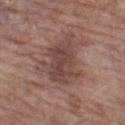Q: Was a biopsy performed?
A: catalogued during a skin exam; not biopsied
Q: Illumination type?
A: white-light illumination
Q: What is the anatomic site?
A: the leg
Q: What is the imaging modality?
A: ~15 mm crop, total-body skin-cancer survey
Q: What is the lesion's diameter?
A: ~5.5 mm (longest diameter)
Q: Patient demographics?
A: female, approximately 70 years of age
Q: What did automated image analysis measure?
A: a lesion area of about 16 mm², an eccentricity of roughly 0.75, and two-axis asymmetry of about 0.35; a lesion color around L≈44 a*≈18 b*≈22 in CIELAB and a lesion–skin lightness drop of about 9; a classifier nevus-likeness of about 0/100